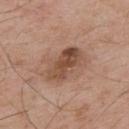{"biopsy_status": "not biopsied; imaged during a skin examination", "lesion_size": {"long_diameter_mm_approx": 5.5}, "site": "upper back", "patient": {"sex": "male", "age_approx": 55}, "image": {"source": "total-body photography crop", "field_of_view_mm": 15}}